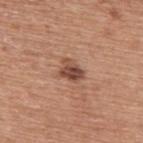Imaged during a routine full-body skin examination; the lesion was not biopsied and no histopathology is available. The lesion is on the upper back. The patient is a male about 75 years old. Cropped from a whole-body photographic skin survey; the tile spans about 15 mm.